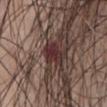Part of a total-body skin-imaging series; this lesion was reviewed on a skin check and was not flagged for biopsy.
The total-body-photography lesion software estimated a shape-asymmetry score of about 0.25 (0 = symmetric). And it measured a mean CIELAB color near L≈31 a*≈20 b*≈17, a lesion–skin lightness drop of about 10, and a lesion-to-skin contrast of about 10 (normalized; higher = more distinct). And it measured a nevus-likeness score of about 20/100.
The lesion's longest dimension is about 3 mm.
A 15 mm crop from a total-body photograph taken for skin-cancer surveillance.
This is a white-light tile.
A male subject, aged approximately 65.
The lesion is on the abdomen.